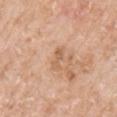The lesion was tiled from a total-body skin photograph and was not biopsied.
From the right upper arm.
About 3 mm across.
The total-body-photography lesion software estimated an area of roughly 2.5 mm² and an eccentricity of roughly 0.95. The software also gave a classifier nevus-likeness of about 0/100 and a detector confidence of about 100 out of 100 that the crop contains a lesion.
A female subject, aged around 75.
A close-up tile cropped from a whole-body skin photograph, about 15 mm across.
This is a white-light tile.